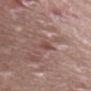{"biopsy_status": "not biopsied; imaged during a skin examination", "lighting": "white-light", "patient": {"sex": "female", "age_approx": 40}, "image": {"source": "total-body photography crop", "field_of_view_mm": 15}, "site": "front of the torso", "lesion_size": {"long_diameter_mm_approx": 2.5}}